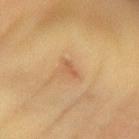The lesion was photographed on a routine skin check and not biopsied; there is no pathology result.
The lesion is on the right forearm.
Automated image analysis of the tile measured an area of roughly 2 mm² and a shape eccentricity near 0.9. The analysis additionally found a mean CIELAB color near L≈58 a*≈22 b*≈38, a lesion–skin lightness drop of about 8, and a normalized border contrast of about 5.5.
The recorded lesion diameter is about 2.5 mm.
This is a cross-polarized tile.
The patient is approximately 60 years of age.
A 15 mm close-up extracted from a 3D total-body photography capture.